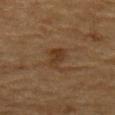Recorded during total-body skin imaging; not selected for excision or biopsy. The tile uses cross-polarized illumination. Located on the upper back. A male patient, approximately 85 years of age or older. Cropped from a total-body skin-imaging series; the visible field is about 15 mm.